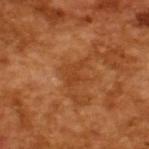workup: catalogued during a skin exam; not biopsied
lesion diameter: about 3 mm
lighting: cross-polarized illumination
acquisition: total-body-photography crop, ~15 mm field of view
patient: male, approximately 65 years of age
TBP lesion metrics: a lesion color around L≈41 a*≈27 b*≈39 in CIELAB, about 6 CIELAB-L* units darker than the surrounding skin, and a lesion-to-skin contrast of about 5 (normalized; higher = more distinct)